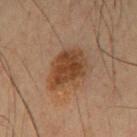Imaged with cross-polarized lighting.
A male patient, aged 48 to 52.
The lesion-visualizer software estimated roughly 8 lightness units darker than nearby skin. The software also gave border irregularity of about 3 on a 0–10 scale, a color-variation rating of about 3.5/10, and radial color variation of about 1.
A lesion tile, about 15 mm wide, cut from a 3D total-body photograph.
Located on the front of the torso.
The lesion's longest dimension is about 5.5 mm.